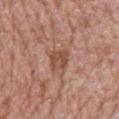Recorded during total-body skin imaging; not selected for excision or biopsy.
On the upper back.
The subject is a female roughly 70 years of age.
This image is a 15 mm lesion crop taken from a total-body photograph.
The tile uses white-light illumination.
Approximately 2.5 mm at its widest.